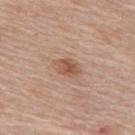<lesion>
<biopsy_status>not biopsied; imaged during a skin examination</biopsy_status>
<image>
  <source>total-body photography crop</source>
  <field_of_view_mm>15</field_of_view_mm>
</image>
<lesion_size>
  <long_diameter_mm_approx>3.0</long_diameter_mm_approx>
</lesion_size>
<site>upper back</site>
<patient>
  <sex>female</sex>
  <age_approx>65</age_approx>
</patient>
</lesion>